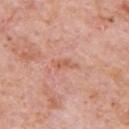From the chest. A male subject in their 80s. A region of skin cropped from a whole-body photographic capture, roughly 15 mm wide.